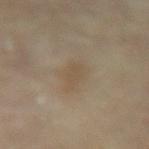Assessment: Captured during whole-body skin photography for melanoma surveillance; the lesion was not biopsied. Clinical summary: The lesion is on the lower back. The patient is a female aged around 60. This is a cross-polarized tile. The recorded lesion diameter is about 3.5 mm. This image is a 15 mm lesion crop taken from a total-body photograph.